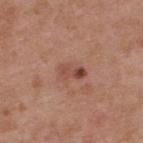Impression: Captured during whole-body skin photography for melanoma surveillance; the lesion was not biopsied. Background: The lesion-visualizer software estimated border irregularity of about 3 on a 0–10 scale. Cropped from a whole-body photographic skin survey; the tile spans about 15 mm. Captured under white-light illumination. A male subject aged 53–57. Longest diameter approximately 3 mm. The lesion is located on the upper back.